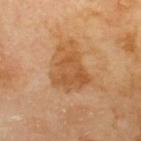Q: Was this lesion biopsied?
A: catalogued during a skin exam; not biopsied
Q: What is the imaging modality?
A: ~15 mm tile from a whole-body skin photo
Q: How was the tile lit?
A: cross-polarized
Q: How large is the lesion?
A: about 5.5 mm
Q: Who is the patient?
A: male, about 70 years old
Q: What is the anatomic site?
A: the upper back
Q: What did automated image analysis measure?
A: a border-irregularity rating of about 5/10, internal color variation of about 3.5 on a 0–10 scale, and a peripheral color-asymmetry measure near 1; an automated nevus-likeness rating near 5 out of 100 and lesion-presence confidence of about 100/100